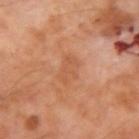Recorded during total-body skin imaging; not selected for excision or biopsy. From the arm. A region of skin cropped from a whole-body photographic capture, roughly 15 mm wide. The subject is a male aged 68–72. The lesion's longest dimension is about 4 mm. The tile uses cross-polarized illumination.